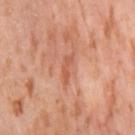Notes:
- notes — no biopsy performed (imaged during a skin exam)